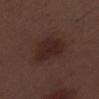Recorded during total-body skin imaging; not selected for excision or biopsy. A region of skin cropped from a whole-body photographic capture, roughly 15 mm wide. On the left lower leg. Automated tile analysis of the lesion measured a lesion color around L≈24 a*≈18 b*≈18 in CIELAB. Measured at roughly 5 mm in maximum diameter. The subject is a male aged approximately 70.